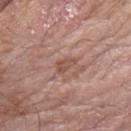biopsy status: imaged on a skin check; not biopsied
patient: male, aged 58–62
tile lighting: white-light illumination
size: about 2.5 mm
automated lesion analysis: a footprint of about 3.5 mm², an outline eccentricity of about 0.85 (0 = round, 1 = elongated), and a symmetry-axis asymmetry near 0.45; an average lesion color of about L≈51 a*≈20 b*≈26 (CIELAB) and a normalized border contrast of about 6.5; a within-lesion color-variation index near 2/10 and peripheral color asymmetry of about 0.5; an automated nevus-likeness rating near 0 out of 100 and a lesion-detection confidence of about 100/100
body site: the left forearm
image: ~15 mm tile from a whole-body skin photo